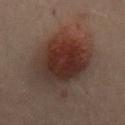Located on the mid back.
A male subject, roughly 50 years of age.
A roughly 15 mm field-of-view crop from a total-body skin photograph.